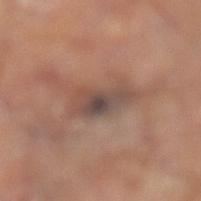Part of a total-body skin-imaging series; this lesion was reviewed on a skin check and was not flagged for biopsy.
Captured under cross-polarized illumination.
Measured at roughly 5.5 mm in maximum diameter.
From the left lower leg.
A 15 mm close-up tile from a total-body photography series done for melanoma screening.
The lesion-visualizer software estimated a lesion area of about 9.5 mm², an outline eccentricity of about 0.9 (0 = round, 1 = elongated), and two-axis asymmetry of about 0.3.